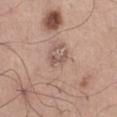| feature | finding |
|---|---|
| patient | male, approximately 55 years of age |
| illumination | white-light |
| automated lesion analysis | a footprint of about 3 mm² and an outline eccentricity of about 0.9 (0 = round, 1 = elongated); roughly 9 lightness units darker than nearby skin |
| anatomic site | the right thigh |
| image | 15 mm crop, total-body photography |